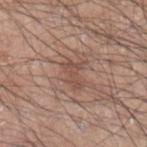{
  "biopsy_status": "not biopsied; imaged during a skin examination",
  "image": {
    "source": "total-body photography crop",
    "field_of_view_mm": 15
  },
  "lighting": "white-light",
  "patient": {
    "sex": "male",
    "age_approx": 70
  },
  "site": "arm",
  "lesion_size": {
    "long_diameter_mm_approx": 3.5
  }
}